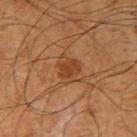<case>
<biopsy_status>not biopsied; imaged during a skin examination</biopsy_status>
</case>